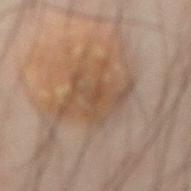{"biopsy_status": "not biopsied; imaged during a skin examination", "lesion_size": {"long_diameter_mm_approx": 5.5}, "patient": {"sex": "male", "age_approx": 50}, "image": {"source": "total-body photography crop", "field_of_view_mm": 15}, "automated_metrics": {"border_irregularity_0_10": 6.5, "color_variation_0_10": 4.0}, "lighting": "cross-polarized", "site": "abdomen"}